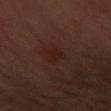| key | value |
|---|---|
| biopsy status | catalogued during a skin exam; not biopsied |
| patient | female, aged 58–62 |
| body site | the front of the torso |
| image source | total-body-photography crop, ~15 mm field of view |